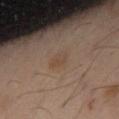follow-up: no biopsy performed (imaged during a skin exam) | lesion diameter: ~2.5 mm (longest diameter) | site: the abdomen | lighting: cross-polarized | acquisition: 15 mm crop, total-body photography | patient: male, aged approximately 50.